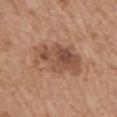Q: Was a biopsy performed?
A: imaged on a skin check; not biopsied
Q: What are the patient's age and sex?
A: male, aged approximately 70
Q: What is the anatomic site?
A: the mid back
Q: What lighting was used for the tile?
A: white-light illumination
Q: Lesion size?
A: ~6.5 mm (longest diameter)
Q: What kind of image is this?
A: 15 mm crop, total-body photography